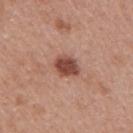– biopsy status · imaged on a skin check; not biopsied